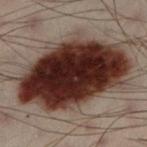Imaged during a routine full-body skin examination; the lesion was not biopsied and no histopathology is available. Imaged with cross-polarized lighting. Located on the left lower leg. A male patient, aged 48–52. A region of skin cropped from a whole-body photographic capture, roughly 15 mm wide.